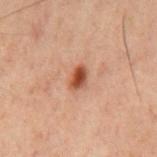location = the mid back; TBP lesion metrics = a footprint of about 4 mm² and a shape eccentricity near 0.75; subject = male, aged around 60; image = 15 mm crop, total-body photography; size = ≈2.5 mm.